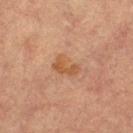Case summary:
– notes — catalogued during a skin exam; not biopsied
– automated metrics — a lesion area of about 5.5 mm², an eccentricity of roughly 0.8, and two-axis asymmetry of about 0.35
– illumination — cross-polarized illumination
– diameter — about 3.5 mm
– image source — 15 mm crop, total-body photography
– patient — female, aged 58 to 62
– site — the left thigh